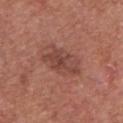workup: catalogued during a skin exam; not biopsied | lesion size: ~5 mm (longest diameter) | automated metrics: a classifier nevus-likeness of about 15/100 and a detector confidence of about 100 out of 100 that the crop contains a lesion | patient: male, aged approximately 75 | anatomic site: the chest | tile lighting: white-light | image source: 15 mm crop, total-body photography.